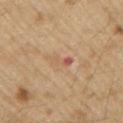Captured during whole-body skin photography for melanoma surveillance; the lesion was not biopsied.
Imaged with white-light lighting.
About 3 mm across.
A region of skin cropped from a whole-body photographic capture, roughly 15 mm wide.
A male patient about 70 years old.
From the right upper arm.
Automated tile analysis of the lesion measured a lesion area of about 3 mm², an outline eccentricity of about 0.9 (0 = round, 1 = elongated), and a shape-asymmetry score of about 0.4 (0 = symmetric). The software also gave a border-irregularity rating of about 4.5/10, a within-lesion color-variation index near 0/10, and radial color variation of about 0. It also reported a classifier nevus-likeness of about 0/100 and lesion-presence confidence of about 100/100.